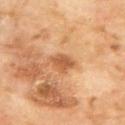| key | value |
|---|---|
| biopsy status | catalogued during a skin exam; not biopsied |
| acquisition | ~15 mm crop, total-body skin-cancer survey |
| subject | male, approximately 70 years of age |
| site | the upper back |